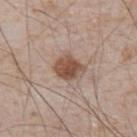follow-up: total-body-photography surveillance lesion; no biopsy | size: ≈3.5 mm | illumination: white-light | body site: the abdomen | subject: male, aged approximately 80 | imaging modality: ~15 mm crop, total-body skin-cancer survey | automated metrics: a lesion–skin lightness drop of about 13 and a normalized lesion–skin contrast near 9.5; an automated nevus-likeness rating near 95 out of 100 and lesion-presence confidence of about 100/100.